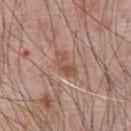notes: catalogued during a skin exam; not biopsied | lesion size: about 3.5 mm | anatomic site: the front of the torso | image: ~15 mm tile from a whole-body skin photo | automated metrics: a border-irregularity index near 6.5/10, a within-lesion color-variation index near 0.5/10, and a peripheral color-asymmetry measure near 0; an automated nevus-likeness rating near 10 out of 100 | illumination: white-light illumination | subject: male, aged 58–62.